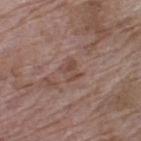Case summary:
- biopsy status: total-body-photography surveillance lesion; no biopsy
- image: ~15 mm crop, total-body skin-cancer survey
- site: the left thigh
- patient: female, in their 70s
- illumination: white-light